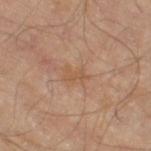Q: What is the lesion's diameter?
A: about 2.5 mm
Q: Automated lesion metrics?
A: two-axis asymmetry of about 0.35; a classifier nevus-likeness of about 0/100 and lesion-presence confidence of about 100/100
Q: Patient demographics?
A: male, in their 70s
Q: How was this image acquired?
A: ~15 mm tile from a whole-body skin photo
Q: Lesion location?
A: the right thigh